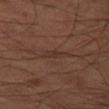The patient is a male in their 60s.
A lesion tile, about 15 mm wide, cut from a 3D total-body photograph.
The lesion is on the left thigh.